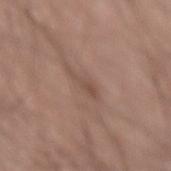{
  "biopsy_status": "not biopsied; imaged during a skin examination",
  "image": {
    "source": "total-body photography crop",
    "field_of_view_mm": 15
  },
  "site": "arm",
  "patient": {
    "sex": "male",
    "age_approx": 55
  },
  "lighting": "white-light"
}